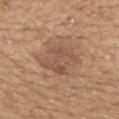{"biopsy_status": "not biopsied; imaged during a skin examination", "image": {"source": "total-body photography crop", "field_of_view_mm": 15}, "patient": {"sex": "female", "age_approx": 70}, "lesion_size": {"long_diameter_mm_approx": 5.5}, "site": "upper back"}